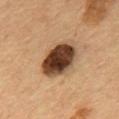Part of a total-body skin-imaging series; this lesion was reviewed on a skin check and was not flagged for biopsy.